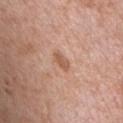Findings:
- notes · total-body-photography surveillance lesion; no biopsy
- patient · male, approximately 65 years of age
- lesion size · ≈2.5 mm
- automated metrics · a lesion area of about 3 mm², an eccentricity of roughly 0.85, and a shape-asymmetry score of about 0.3 (0 = symmetric); a lesion color around L≈57 a*≈22 b*≈31 in CIELAB, about 9 CIELAB-L* units darker than the surrounding skin, and a normalized lesion–skin contrast near 6.5
- image source · total-body-photography crop, ~15 mm field of view
- site · the chest